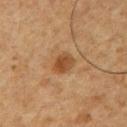Findings:
* follow-up: imaged on a skin check; not biopsied
* patient: male, about 60 years old
* image source: 15 mm crop, total-body photography
* automated lesion analysis: an area of roughly 5 mm² and an eccentricity of roughly 0.6; a lesion color around L≈39 a*≈18 b*≈32 in CIELAB, a lesion–skin lightness drop of about 9, and a normalized border contrast of about 8; an automated nevus-likeness rating near 60 out of 100 and lesion-presence confidence of about 100/100
* body site: the chest